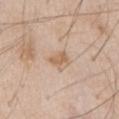{"biopsy_status": "not biopsied; imaged during a skin examination", "lighting": "white-light", "image": {"source": "total-body photography crop", "field_of_view_mm": 15}, "site": "abdomen", "patient": {"sex": "male", "age_approx": 60}, "lesion_size": {"long_diameter_mm_approx": 2.5}, "automated_metrics": {"cielab_L": 62, "cielab_a": 17, "cielab_b": 32, "vs_skin_darker_L": 9.0, "nevus_likeness_0_100": 10, "lesion_detection_confidence_0_100": 100}}